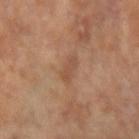Clinical impression:
The lesion was photographed on a routine skin check and not biopsied; there is no pathology result.
Background:
Measured at roughly 3 mm in maximum diameter. The patient is a female approximately 65 years of age. Located on the left arm. A lesion tile, about 15 mm wide, cut from a 3D total-body photograph. This is a cross-polarized tile.